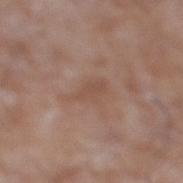Notes:
- notes · no biopsy performed (imaged during a skin exam)
- location · the right lower leg
- diameter · ≈4 mm
- patient · male, aged approximately 60
- image · ~15 mm tile from a whole-body skin photo
- tile lighting · white-light